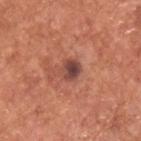The lesion's longest dimension is about 2.5 mm.
A male patient aged 63–67.
The lesion-visualizer software estimated roughly 12 lightness units darker than nearby skin and a normalized lesion–skin contrast near 9.5. The software also gave lesion-presence confidence of about 100/100.
A 15 mm crop from a total-body photograph taken for skin-cancer surveillance.
The lesion is on the upper back.